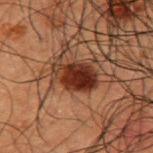Assessment: The lesion was tiled from a total-body skin photograph and was not biopsied. Context: Located on the upper back. Measured at roughly 5 mm in maximum diameter. A 15 mm crop from a total-body photograph taken for skin-cancer surveillance. This is a cross-polarized tile. The subject is a male aged 48–52.